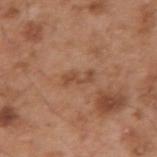Q: Is there a histopathology result?
A: imaged on a skin check; not biopsied
Q: What is the anatomic site?
A: the arm
Q: How was this image acquired?
A: total-body-photography crop, ~15 mm field of view
Q: Lesion size?
A: ~3 mm (longest diameter)
Q: Patient demographics?
A: male, aged 53–57
Q: Illumination type?
A: white-light